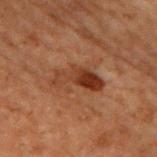Recorded during total-body skin imaging; not selected for excision or biopsy. Cropped from a whole-body photographic skin survey; the tile spans about 15 mm. Imaged with cross-polarized lighting. Automated image analysis of the tile measured an area of roughly 9.5 mm², an outline eccentricity of about 0.9 (0 = round, 1 = elongated), and a symmetry-axis asymmetry near 0.45. The analysis additionally found a border-irregularity rating of about 5.5/10. The lesion is on the back. A male patient, in their 70s. Longest diameter approximately 5.5 mm.